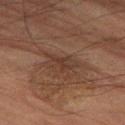This lesion was catalogued during total-body skin photography and was not selected for biopsy. Imaged with cross-polarized lighting. The lesion-visualizer software estimated a border-irregularity rating of about 7/10, internal color variation of about 0 on a 0–10 scale, and radial color variation of about 0. And it measured a nevus-likeness score of about 0/100 and lesion-presence confidence of about 80/100. A 15 mm crop from a total-body photograph taken for skin-cancer surveillance. A male subject, aged around 85 or older. The lesion is on the left thigh.